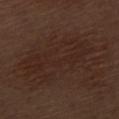{
  "biopsy_status": "not biopsied; imaged during a skin examination",
  "automated_metrics": {
    "vs_skin_darker_L": 4.0,
    "vs_skin_contrast_norm": 5.0,
    "border_irregularity_0_10": 8.0,
    "peripheral_color_asymmetry": 1.0,
    "nevus_likeness_0_100": 0,
    "lesion_detection_confidence_0_100": 100
  },
  "image": {
    "source": "total-body photography crop",
    "field_of_view_mm": 15
  },
  "site": "left thigh",
  "lighting": "white-light",
  "lesion_size": {
    "long_diameter_mm_approx": 9.5
  },
  "patient": {
    "sex": "male",
    "age_approx": 70
  }
}